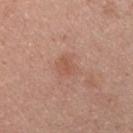{
  "biopsy_status": "not biopsied; imaged during a skin examination",
  "automated_metrics": {
    "area_mm2_approx": 4.5,
    "eccentricity": 0.55,
    "shape_asymmetry": 0.2,
    "cielab_L": 55,
    "cielab_a": 23,
    "cielab_b": 29,
    "vs_skin_darker_L": 6.0,
    "vs_skin_contrast_norm": 5.0,
    "border_irregularity_0_10": 2.0,
    "color_variation_0_10": 2.0,
    "peripheral_color_asymmetry": 0.5,
    "nevus_likeness_0_100": 5
  },
  "site": "chest",
  "lighting": "white-light",
  "patient": {
    "sex": "male",
    "age_approx": 25
  },
  "image": {
    "source": "total-body photography crop",
    "field_of_view_mm": 15
  },
  "lesion_size": {
    "long_diameter_mm_approx": 2.5
  }
}